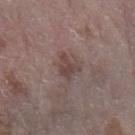Case summary:
- workup — no biopsy performed (imaged during a skin exam)
- automated metrics — a mean CIELAB color near L≈43 a*≈16 b*≈19 and roughly 8 lightness units darker than nearby skin; a border-irregularity index near 4/10, a within-lesion color-variation index near 2.5/10, and radial color variation of about 1
- subject — female, about 65 years old
- image source — 15 mm crop, total-body photography
- lesion diameter — ≈2.5 mm
- body site — the left forearm